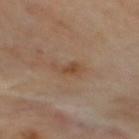Q: Was a biopsy performed?
A: no biopsy performed (imaged during a skin exam)
Q: How was this image acquired?
A: ~15 mm tile from a whole-body skin photo
Q: Where on the body is the lesion?
A: the mid back
Q: Illumination type?
A: cross-polarized illumination